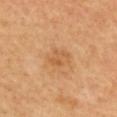Clinical impression: This lesion was catalogued during total-body skin photography and was not selected for biopsy. Background: A female subject, in their 60s. Measured at roughly 3 mm in maximum diameter. The lesion is on the upper back. A lesion tile, about 15 mm wide, cut from a 3D total-body photograph.